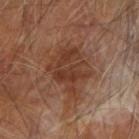workup: no biopsy performed (imaged during a skin exam)
diameter: ≈6 mm
acquisition: 15 mm crop, total-body photography
illumination: cross-polarized
patient: male, aged 68 to 72
automated lesion analysis: an area of roughly 18 mm² and a symmetry-axis asymmetry near 0.25; a border-irregularity index near 3.5/10, a color-variation rating of about 4.5/10, and peripheral color asymmetry of about 1.5
anatomic site: the arm